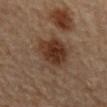Imaged during a routine full-body skin examination; the lesion was not biopsied and no histopathology is available.
Imaged with cross-polarized lighting.
A 15 mm crop from a total-body photograph taken for skin-cancer surveillance.
An algorithmic analysis of the crop reported a lesion area of about 20 mm², a shape eccentricity near 0.75, and a shape-asymmetry score of about 0.25 (0 = symmetric). It also reported a lesion color around L≈35 a*≈17 b*≈26 in CIELAB and about 10 CIELAB-L* units darker than the surrounding skin.
Located on the mid back.
The subject is a male approximately 85 years of age or older.